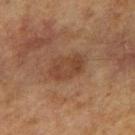Q: Is there a histopathology result?
A: total-body-photography surveillance lesion; no biopsy
Q: What lighting was used for the tile?
A: cross-polarized illumination
Q: Automated lesion metrics?
A: an area of roughly 10 mm² and an outline eccentricity of about 0.8 (0 = round, 1 = elongated); a border-irregularity index near 2/10, a color-variation rating of about 2.5/10, and peripheral color asymmetry of about 1
Q: What is the anatomic site?
A: the arm
Q: How large is the lesion?
A: about 4.5 mm
Q: What kind of image is this?
A: ~15 mm tile from a whole-body skin photo
Q: Who is the patient?
A: male, approximately 65 years of age